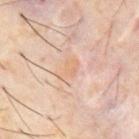| feature | finding |
|---|---|
| workup | catalogued during a skin exam; not biopsied |
| patient | male, approximately 60 years of age |
| size | ~3 mm (longest diameter) |
| image | ~15 mm crop, total-body skin-cancer survey |
| body site | the abdomen |
| automated metrics | a footprint of about 4 mm² and a shape eccentricity near 0.8; a lesion color around L≈65 a*≈18 b*≈32 in CIELAB, about 5 CIELAB-L* units darker than the surrounding skin, and a normalized lesion–skin contrast near 5 |